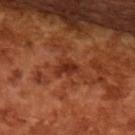A male subject aged approximately 65. Imaged with cross-polarized lighting. A 15 mm close-up extracted from a 3D total-body photography capture.